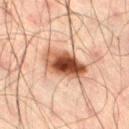No biopsy was performed on this lesion — it was imaged during a full skin examination and was not determined to be concerning. A male patient approximately 50 years of age. Captured under cross-polarized illumination. The lesion-visualizer software estimated an eccentricity of roughly 0.75 and a shape-asymmetry score of about 0.15 (0 = symmetric). The analysis additionally found a mean CIELAB color near L≈42 a*≈20 b*≈27. And it measured a border-irregularity rating of about 2.5/10, a color-variation rating of about 10/10, and peripheral color asymmetry of about 3.5. Cropped from a total-body skin-imaging series; the visible field is about 15 mm. Approximately 5.5 mm at its widest. On the right thigh.